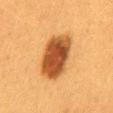* follow-up — imaged on a skin check; not biopsied
* diameter — ≈6.5 mm
* patient — female, in their 40s
* image-analysis metrics — an area of roughly 18 mm², an eccentricity of roughly 0.85, and two-axis asymmetry of about 0.2; a lesion color around L≈42 a*≈23 b*≈37 in CIELAB and about 17 CIELAB-L* units darker than the surrounding skin; border irregularity of about 2 on a 0–10 scale, a color-variation rating of about 5/10, and peripheral color asymmetry of about 1; a nevus-likeness score of about 100/100 and a detector confidence of about 100 out of 100 that the crop contains a lesion
* acquisition — total-body-photography crop, ~15 mm field of view
* site — the mid back
* illumination — cross-polarized illumination